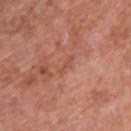workup=catalogued during a skin exam; not biopsied
body site=the chest
patient=male, aged approximately 70
imaging modality=15 mm crop, total-body photography